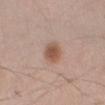Imaged during a routine full-body skin examination; the lesion was not biopsied and no histopathology is available. The subject is a male aged 43–47. Measured at roughly 2.5 mm in maximum diameter. The lesion is on the right forearm. Cropped from a total-body skin-imaging series; the visible field is about 15 mm.